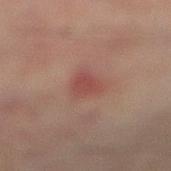follow-up — total-body-photography surveillance lesion; no biopsy.